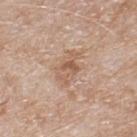The lesion was tiled from a total-body skin photograph and was not biopsied. The lesion-visualizer software estimated a footprint of about 7 mm² and two-axis asymmetry of about 0.35. The software also gave a color-variation rating of about 5.5/10 and radial color variation of about 2. And it measured lesion-presence confidence of about 100/100. Approximately 4 mm at its widest. A 15 mm close-up extracted from a 3D total-body photography capture. The patient is a male aged approximately 80. Imaged with white-light lighting. From the back.